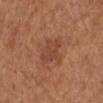workup: total-body-photography surveillance lesion; no biopsy | body site: the left leg | patient: female, aged 63 to 67 | image source: ~15 mm crop, total-body skin-cancer survey.